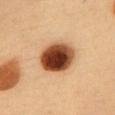Clinical impression: Captured during whole-body skin photography for melanoma surveillance; the lesion was not biopsied. Clinical summary: The lesion is located on the chest. A female subject in their mid- to late 30s. Approximately 5 mm at its widest. A close-up tile cropped from a whole-body skin photograph, about 15 mm across. Captured under cross-polarized illumination.